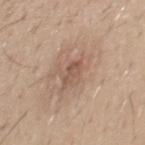{"lesion_size": {"long_diameter_mm_approx": 3.0}, "site": "back", "lighting": "white-light", "automated_metrics": {"cielab_L": 53, "cielab_a": 19, "cielab_b": 27, "vs_skin_darker_L": 9.0, "vs_skin_contrast_norm": 6.0, "border_irregularity_0_10": 3.5, "color_variation_0_10": 1.0, "peripheral_color_asymmetry": 0.5, "nevus_likeness_0_100": 0, "lesion_detection_confidence_0_100": 100}, "patient": {"sex": "male", "age_approx": 40}, "image": {"source": "total-body photography crop", "field_of_view_mm": 15}}